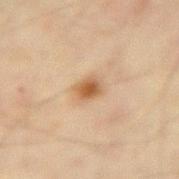Notes:
• workup — no biopsy performed (imaged during a skin exam)
• lighting — cross-polarized illumination
• lesion diameter — about 2.5 mm
• TBP lesion metrics — a footprint of about 4.5 mm² and a symmetry-axis asymmetry near 0.2; a lesion color around L≈50 a*≈17 b*≈33 in CIELAB, a lesion–skin lightness drop of about 11, and a normalized border contrast of about 8.5; a border-irregularity index near 2/10 and a peripheral color-asymmetry measure near 1; an automated nevus-likeness rating near 95 out of 100 and a detector confidence of about 100 out of 100 that the crop contains a lesion
• body site — the right forearm
• image — 15 mm crop, total-body photography
• patient — male, in their mid-40s